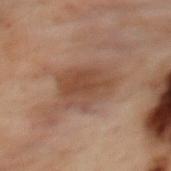Clinical impression:
This lesion was catalogued during total-body skin photography and was not selected for biopsy.
Acquisition and patient details:
Automated tile analysis of the lesion measured a mean CIELAB color near L≈38 a*≈17 b*≈25 and roughly 8 lightness units darker than nearby skin. The analysis additionally found a color-variation rating of about 3.5/10 and peripheral color asymmetry of about 1. The software also gave a nevus-likeness score of about 10/100 and lesion-presence confidence of about 100/100. The lesion is located on the upper back. The subject is a female roughly 55 years of age. A region of skin cropped from a whole-body photographic capture, roughly 15 mm wide. Captured under cross-polarized illumination.